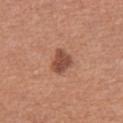biopsy status: total-body-photography surveillance lesion; no biopsy | imaging modality: 15 mm crop, total-body photography | site: the left thigh | diameter: about 3 mm | TBP lesion metrics: an average lesion color of about L≈48 a*≈24 b*≈29 (CIELAB), a lesion–skin lightness drop of about 12, and a lesion-to-skin contrast of about 9 (normalized; higher = more distinct); a detector confidence of about 100 out of 100 that the crop contains a lesion | patient: female, in their 60s.